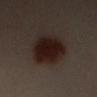The lesion was tiled from a total-body skin photograph and was not biopsied. The patient is a female aged 38 to 42. About 7 mm across. Imaged with cross-polarized lighting. A lesion tile, about 15 mm wide, cut from a 3D total-body photograph. Located on the left arm. Automated image analysis of the tile measured a shape eccentricity near 0.85 and a symmetry-axis asymmetry near 0.15. It also reported a classifier nevus-likeness of about 100/100 and a lesion-detection confidence of about 100/100.